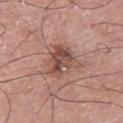biopsy status: catalogued during a skin exam; not biopsied
image: 15 mm crop, total-body photography
automated metrics: a lesion area of about 12 mm² and a shape-asymmetry score of about 0.35 (0 = symmetric); a border-irregularity index near 4/10 and radial color variation of about 2.5; a nevus-likeness score of about 10/100 and a lesion-detection confidence of about 100/100
subject: male, aged 73 to 77
body site: the right thigh